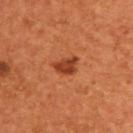Findings:
- patient — male, about 55 years old
- diameter — ~3 mm (longest diameter)
- location — the back
- acquisition — 15 mm crop, total-body photography
- automated metrics — a lesion area of about 4.5 mm² and a symmetry-axis asymmetry near 0.35; border irregularity of about 3.5 on a 0–10 scale and a color-variation rating of about 1.5/10
- illumination — cross-polarized illumination